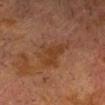No biopsy was performed on this lesion — it was imaged during a full skin examination and was not determined to be concerning. Imaged with cross-polarized lighting. A 15 mm close-up tile from a total-body photography series done for melanoma screening. The patient is a male aged around 75. Longest diameter approximately 5.5 mm. On the head or neck.